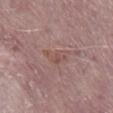The total-body-photography lesion software estimated a mean CIELAB color near L≈51 a*≈20 b*≈22 and roughly 5 lightness units darker than nearby skin. The analysis additionally found border irregularity of about 4.5 on a 0–10 scale, internal color variation of about 2 on a 0–10 scale, and a peripheral color-asymmetry measure near 0.5. It also reported an automated nevus-likeness rating near 0 out of 100.
The lesion is located on the leg.
A 15 mm close-up extracted from a 3D total-body photography capture.
A male patient aged 73–77.